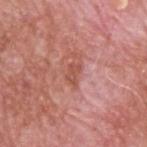workup — total-body-photography surveillance lesion; no biopsy
image — ~15 mm tile from a whole-body skin photo
lesion diameter — ≈2.5 mm
patient — male, in their 60s
body site — the upper back